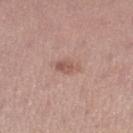Captured during whole-body skin photography for melanoma surveillance; the lesion was not biopsied.
A female subject, aged around 20.
Imaged with white-light lighting.
A close-up tile cropped from a whole-body skin photograph, about 15 mm across.
On the left lower leg.